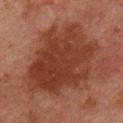Captured during whole-body skin photography for melanoma surveillance; the lesion was not biopsied. The tile uses cross-polarized illumination. A lesion tile, about 15 mm wide, cut from a 3D total-body photograph. On the chest. Automated tile analysis of the lesion measured an average lesion color of about L≈28 a*≈20 b*≈23 (CIELAB), a lesion–skin lightness drop of about 8, and a normalized border contrast of about 9. And it measured border irregularity of about 4 on a 0–10 scale and peripheral color asymmetry of about 1. The software also gave a classifier nevus-likeness of about 5/100 and a detector confidence of about 100 out of 100 that the crop contains a lesion. A male subject roughly 60 years of age. Measured at roughly 10 mm in maximum diameter.